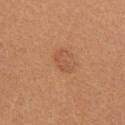No biopsy was performed on this lesion — it was imaged during a full skin examination and was not determined to be concerning.
Cropped from a total-body skin-imaging series; the visible field is about 15 mm.
The lesion is on the arm.
A female subject, about 25 years old.